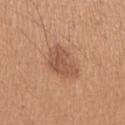Q: Was this lesion biopsied?
A: imaged on a skin check; not biopsied
Q: Where on the body is the lesion?
A: the left upper arm
Q: How was the tile lit?
A: white-light illumination
Q: What are the patient's age and sex?
A: female, aged 38–42
Q: What did automated image analysis measure?
A: a border-irregularity rating of about 3/10
Q: What kind of image is this?
A: ~15 mm crop, total-body skin-cancer survey
Q: Lesion size?
A: ~4.5 mm (longest diameter)